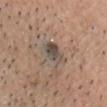Recorded during total-body skin imaging; not selected for excision or biopsy.
On the head or neck.
A male patient about 40 years old.
A 15 mm crop from a total-body photograph taken for skin-cancer surveillance.
Automated tile analysis of the lesion measured a border-irregularity rating of about 2.5/10 and radial color variation of about 2.